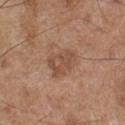The lesion was tiled from a total-body skin photograph and was not biopsied.
A male patient, in their mid-50s.
The recorded lesion diameter is about 4 mm.
Automated image analysis of the tile measured a shape eccentricity near 0.65 and two-axis asymmetry of about 0.3. The software also gave an average lesion color of about L≈50 a*≈19 b*≈30 (CIELAB) and a normalized lesion–skin contrast near 6. And it measured a nevus-likeness score of about 5/100 and lesion-presence confidence of about 100/100.
A roughly 15 mm field-of-view crop from a total-body skin photograph.
From the chest.